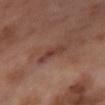The lesion was tiled from a total-body skin photograph and was not biopsied. A close-up tile cropped from a whole-body skin photograph, about 15 mm across. On the leg. A female subject, about 55 years old. The recorded lesion diameter is about 4 mm. This is a cross-polarized tile.